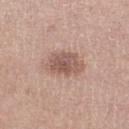{
  "biopsy_status": "not biopsied; imaged during a skin examination",
  "patient": {
    "sex": "female",
    "age_approx": 50
  },
  "site": "left lower leg",
  "image": {
    "source": "total-body photography crop",
    "field_of_view_mm": 15
  }
}